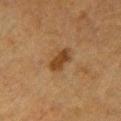The lesion was tiled from a total-body skin photograph and was not biopsied. A 15 mm crop from a total-body photograph taken for skin-cancer surveillance. A female subject approximately 55 years of age. About 3.5 mm across. From the chest. The lesion-visualizer software estimated an area of roughly 5.5 mm² and a shape eccentricity near 0.85. The analysis additionally found a detector confidence of about 100 out of 100 that the crop contains a lesion.